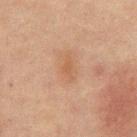The lesion was tiled from a total-body skin photograph and was not biopsied.
The lesion is on the back.
A male subject aged 63–67.
Approximately 3 mm at its widest.
A roughly 15 mm field-of-view crop from a total-body skin photograph.
Imaged with cross-polarized lighting.
The lesion-visualizer software estimated a lesion area of about 3 mm², an eccentricity of roughly 0.9, and a symmetry-axis asymmetry near 0.4. The analysis additionally found an average lesion color of about L≈45 a*≈18 b*≈29 (CIELAB), about 5 CIELAB-L* units darker than the surrounding skin, and a normalized lesion–skin contrast near 5. The software also gave a border-irregularity index near 4.5/10, a within-lesion color-variation index near 0.5/10, and radial color variation of about 0.5.